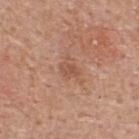Recorded during total-body skin imaging; not selected for excision or biopsy. The patient is a male roughly 55 years of age. Located on the upper back. A lesion tile, about 15 mm wide, cut from a 3D total-body photograph.